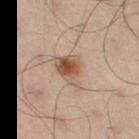Part of a total-body skin-imaging series; this lesion was reviewed on a skin check and was not flagged for biopsy. About 5 mm across. Captured under cross-polarized illumination. A 15 mm close-up extracted from a 3D total-body photography capture. From the lower back. A male patient aged 48–52.